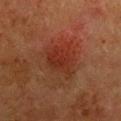{"biopsy_status": "not biopsied; imaged during a skin examination", "lesion_size": {"long_diameter_mm_approx": 4.5}, "patient": {"sex": "female", "age_approx": 50}, "site": "chest", "image": {"source": "total-body photography crop", "field_of_view_mm": 15}}